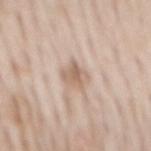Q: What kind of image is this?
A: ~15 mm tile from a whole-body skin photo
Q: Who is the patient?
A: male, aged 63 to 67
Q: Where on the body is the lesion?
A: the mid back